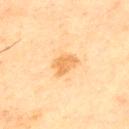Q: Is there a histopathology result?
A: no biopsy performed (imaged during a skin exam)
Q: What lighting was used for the tile?
A: cross-polarized illumination
Q: Automated lesion metrics?
A: a footprint of about 5.5 mm², an outline eccentricity of about 0.7 (0 = round, 1 = elongated), and two-axis asymmetry of about 0.35; a border-irregularity rating of about 3.5/10, internal color variation of about 2 on a 0–10 scale, and a peripheral color-asymmetry measure near 0.5; a classifier nevus-likeness of about 20/100 and a detector confidence of about 100 out of 100 that the crop contains a lesion
Q: Patient demographics?
A: male, aged 43 to 47
Q: How was this image acquired?
A: total-body-photography crop, ~15 mm field of view
Q: Where on the body is the lesion?
A: the upper back